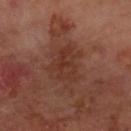– workup — imaged on a skin check; not biopsied
– illumination — cross-polarized
– acquisition — ~15 mm tile from a whole-body skin photo
– patient — male, aged approximately 70
– body site — the right forearm